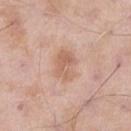<lesion>
  <biopsy_status>not biopsied; imaged during a skin examination</biopsy_status>
  <image>
    <source>total-body photography crop</source>
    <field_of_view_mm>15</field_of_view_mm>
  </image>
  <site>left thigh</site>
  <lighting>white-light</lighting>
  <patient>
    <sex>male</sex>
    <age_approx>55</age_approx>
  </patient>
  <lesion_size>
    <long_diameter_mm_approx>4.0</long_diameter_mm_approx>
  </lesion_size>
</lesion>